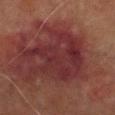Clinical impression:
Captured during whole-body skin photography for melanoma surveillance; the lesion was not biopsied.
Image and clinical context:
The total-body-photography lesion software estimated an area of roughly 32 mm², an eccentricity of roughly 0.7, and a symmetry-axis asymmetry near 0.45. The software also gave border irregularity of about 8.5 on a 0–10 scale, a within-lesion color-variation index near 3.5/10, and a peripheral color-asymmetry measure near 1. The software also gave an automated nevus-likeness rating near 0 out of 100 and a detector confidence of about 75 out of 100 that the crop contains a lesion. Cropped from a total-body skin-imaging series; the visible field is about 15 mm. On the left lower leg. The subject is a male in their 70s. Measured at roughly 8 mm in maximum diameter. The tile uses cross-polarized illumination.